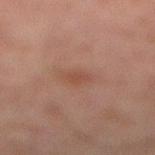The lesion was photographed on a routine skin check and not biopsied; there is no pathology result. Cropped from a whole-body photographic skin survey; the tile spans about 15 mm. On the right lower leg. Imaged with cross-polarized lighting. The lesion's longest dimension is about 2.5 mm. A male patient roughly 30 years of age.